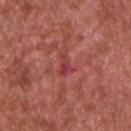No biopsy was performed on this lesion — it was imaged during a full skin examination and was not determined to be concerning.
A lesion tile, about 15 mm wide, cut from a 3D total-body photograph.
A male patient approximately 65 years of age.
Automated tile analysis of the lesion measured an area of roughly 3 mm², an outline eccentricity of about 0.85 (0 = round, 1 = elongated), and two-axis asymmetry of about 0.55. The software also gave border irregularity of about 5.5 on a 0–10 scale and peripheral color asymmetry of about 0. It also reported an automated nevus-likeness rating near 0 out of 100 and lesion-presence confidence of about 95/100.
The lesion's longest dimension is about 2.5 mm.
The lesion is located on the chest.
This is a white-light tile.